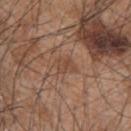{
  "biopsy_status": "not biopsied; imaged during a skin examination",
  "patient": {
    "sex": "male",
    "age_approx": 45
  },
  "image": {
    "source": "total-body photography crop",
    "field_of_view_mm": 15
  },
  "lesion_size": {
    "long_diameter_mm_approx": 2.5
  },
  "site": "upper back"
}